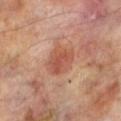notes: no biopsy performed (imaged during a skin exam) | diameter: ~3.5 mm (longest diameter) | lighting: cross-polarized | acquisition: 15 mm crop, total-body photography | subject: male, about 70 years old | body site: the left lower leg | automated lesion analysis: a lesion area of about 9 mm² and an outline eccentricity of about 0.45 (0 = round, 1 = elongated); a lesion color around L≈52 a*≈26 b*≈31 in CIELAB and a lesion–skin lightness drop of about 9; radial color variation of about 1.